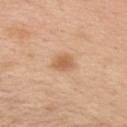Q: Is there a histopathology result?
A: no biopsy performed (imaged during a skin exam)
Q: How was this image acquired?
A: total-body-photography crop, ~15 mm field of view
Q: What is the lesion's diameter?
A: ~2.5 mm (longest diameter)
Q: Where on the body is the lesion?
A: the back
Q: What are the patient's age and sex?
A: male, in their mid-50s
Q: How was the tile lit?
A: white-light
Q: Automated lesion metrics?
A: a lesion area of about 4.5 mm², an eccentricity of roughly 0.6, and a shape-asymmetry score of about 0.2 (0 = symmetric); a lesion-detection confidence of about 100/100